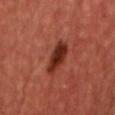<case>
<biopsy_status>not biopsied; imaged during a skin examination</biopsy_status>
<lighting>cross-polarized</lighting>
<automated_metrics>
  <cielab_L>23</cielab_L>
  <cielab_a>23</cielab_a>
  <cielab_b>24</cielab_b>
  <vs_skin_darker_L>11.0</vs_skin_darker_L>
  <vs_skin_contrast_norm>11.0</vs_skin_contrast_norm>
  <border_irregularity_0_10>3.5</border_irregularity_0_10>
  <color_variation_0_10>3.0</color_variation_0_10>
  <peripheral_color_asymmetry>1.0</peripheral_color_asymmetry>
</automated_metrics>
<patient>
  <sex>male</sex>
  <age_approx>60</age_approx>
</patient>
<lesion_size>
  <long_diameter_mm_approx>5.0</long_diameter_mm_approx>
</lesion_size>
<site>chest</site>
<image>
  <source>total-body photography crop</source>
  <field_of_view_mm>15</field_of_view_mm>
</image>
</case>